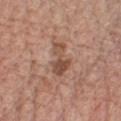This lesion was catalogued during total-body skin photography and was not selected for biopsy. Cropped from a whole-body photographic skin survey; the tile spans about 15 mm. Located on the chest. A male patient about 60 years old. An algorithmic analysis of the crop reported an outline eccentricity of about 0.85 (0 = round, 1 = elongated) and a shape-asymmetry score of about 0.45 (0 = symmetric). The software also gave a mean CIELAB color near L≈50 a*≈21 b*≈28, a lesion–skin lightness drop of about 10, and a lesion-to-skin contrast of about 7 (normalized; higher = more distinct). It also reported an automated nevus-likeness rating near 0 out of 100 and lesion-presence confidence of about 100/100. Measured at roughly 4 mm in maximum diameter.